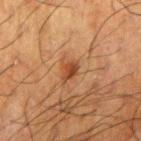biopsy status: catalogued during a skin exam; not biopsied | lesion diameter: ~2.5 mm (longest diameter) | tile lighting: cross-polarized illumination | imaging modality: total-body-photography crop, ~15 mm field of view | anatomic site: the arm | subject: male, in their 60s.